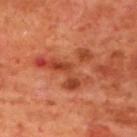Imaged during a routine full-body skin examination; the lesion was not biopsied and no histopathology is available. A male patient, aged 68 to 72. On the mid back. A 15 mm crop from a total-body photograph taken for skin-cancer surveillance.